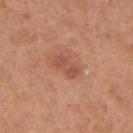Part of a total-body skin-imaging series; this lesion was reviewed on a skin check and was not flagged for biopsy. On the right upper arm. A 15 mm close-up extracted from a 3D total-body photography capture. A female patient, aged around 40.